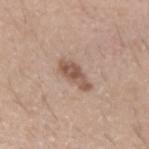<tbp_lesion>
  <biopsy_status>not biopsied; imaged during a skin examination</biopsy_status>
  <lesion_size>
    <long_diameter_mm_approx>4.5</long_diameter_mm_approx>
  </lesion_size>
  <patient>
    <sex>male</sex>
    <age_approx>40</age_approx>
  </patient>
  <automated_metrics>
    <area_mm2_approx>6.5</area_mm2_approx>
    <eccentricity>0.9</eccentricity>
    <shape_asymmetry>0.25</shape_asymmetry>
    <cielab_L>54</cielab_L>
    <cielab_a>18</cielab_a>
    <cielab_b>26</cielab_b>
    <vs_skin_darker_L>12.0</vs_skin_darker_L>
    <vs_skin_contrast_norm>8.0</vs_skin_contrast_norm>
    <border_irregularity_0_10>3.0</border_irregularity_0_10>
    <color_variation_0_10>4.0</color_variation_0_10>
    <peripheral_color_asymmetry>1.5</peripheral_color_asymmetry>
  </automated_metrics>
  <site>left forearm</site>
  <image>
    <source>total-body photography crop</source>
    <field_of_view_mm>15</field_of_view_mm>
  </image>
</tbp_lesion>